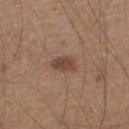biopsy_status: not biopsied; imaged during a skin examination
lighting: white-light
patient:
  sex: male
  age_approx: 65
site: right lower leg
lesion_size:
  long_diameter_mm_approx: 3.0
image:
  source: total-body photography crop
  field_of_view_mm: 15